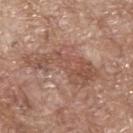This lesion was catalogued during total-body skin photography and was not selected for biopsy.
Automated tile analysis of the lesion measured an average lesion color of about L≈51 a*≈21 b*≈27 (CIELAB), roughly 9 lightness units darker than nearby skin, and a normalized lesion–skin contrast near 6.5.
Imaged with white-light lighting.
A male subject aged 68–72.
Cropped from a total-body skin-imaging series; the visible field is about 15 mm.
From the upper back.